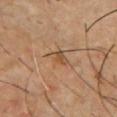Q: Was this lesion biopsied?
A: no biopsy performed (imaged during a skin exam)
Q: Where on the body is the lesion?
A: the chest
Q: What is the imaging modality?
A: total-body-photography crop, ~15 mm field of view
Q: Lesion size?
A: about 3.5 mm
Q: What lighting was used for the tile?
A: cross-polarized illumination
Q: Automated lesion metrics?
A: a peripheral color-asymmetry measure near 1
Q: Patient demographics?
A: male, aged 53–57